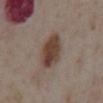{"biopsy_status": "not biopsied; imaged during a skin examination", "site": "abdomen", "patient": {"sex": "male", "age_approx": 75}, "image": {"source": "total-body photography crop", "field_of_view_mm": 15}}